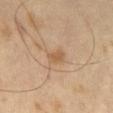Q: Was this lesion biopsied?
A: no biopsy performed (imaged during a skin exam)
Q: Who is the patient?
A: male, aged 63–67
Q: How large is the lesion?
A: about 2.5 mm
Q: What kind of image is this?
A: 15 mm crop, total-body photography
Q: What did automated image analysis measure?
A: a footprint of about 3.5 mm², an outline eccentricity of about 0.8 (0 = round, 1 = elongated), and two-axis asymmetry of about 0.25; internal color variation of about 1 on a 0–10 scale and peripheral color asymmetry of about 0.5